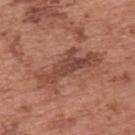{
  "biopsy_status": "not biopsied; imaged during a skin examination",
  "lesion_size": {
    "long_diameter_mm_approx": 7.5
  },
  "lighting": "white-light",
  "site": "upper back",
  "image": {
    "source": "total-body photography crop",
    "field_of_view_mm": 15
  },
  "patient": {
    "sex": "male",
    "age_approx": 70
  }
}